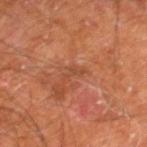<case>
<biopsy_status>not biopsied; imaged during a skin examination</biopsy_status>
<lesion_size>
  <long_diameter_mm_approx>2.5</long_diameter_mm_approx>
</lesion_size>
<automated_metrics>
  <nevus_likeness_0_100>0</nevus_likeness_0_100>
  <lesion_detection_confidence_0_100>100</lesion_detection_confidence_0_100>
</automated_metrics>
<image>
  <source>total-body photography crop</source>
  <field_of_view_mm>15</field_of_view_mm>
</image>
<lighting>cross-polarized</lighting>
<patient>
  <sex>male</sex>
  <age_approx>60</age_approx>
</patient>
<site>leg</site>
</case>